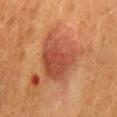{"biopsy_status": "not biopsied; imaged during a skin examination", "patient": {"sex": "female", "age_approx": 50}, "lighting": "cross-polarized", "automated_metrics": {"area_mm2_approx": 23.0, "eccentricity": 0.7, "shape_asymmetry": 0.3, "border_irregularity_0_10": 3.5, "color_variation_0_10": 4.5, "nevus_likeness_0_100": 20, "lesion_detection_confidence_0_100": 100}, "lesion_size": {"long_diameter_mm_approx": 7.0}, "image": {"source": "total-body photography crop", "field_of_view_mm": 15}, "site": "mid back"}